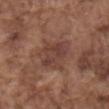follow-up=no biopsy performed (imaged during a skin exam)
anatomic site=the mid back
size=~4.5 mm (longest diameter)
image=total-body-photography crop, ~15 mm field of view
subject=male, roughly 75 years of age
automated lesion analysis=a mean CIELAB color near L≈41 a*≈20 b*≈24 and a normalized lesion–skin contrast near 6.5; border irregularity of about 3 on a 0–10 scale, a within-lesion color-variation index near 3.5/10, and peripheral color asymmetry of about 1; a classifier nevus-likeness of about 5/100 and lesion-presence confidence of about 100/100
tile lighting=white-light